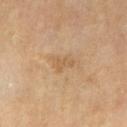- workup — catalogued during a skin exam; not biopsied
- subject — male, in their mid-60s
- imaging modality — ~15 mm tile from a whole-body skin photo
- location — the leg
- diameter — about 2.5 mm